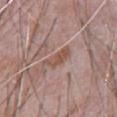notes: catalogued during a skin exam; not biopsied
patient: male, aged 68–72
body site: the chest
image: ~15 mm crop, total-body skin-cancer survey